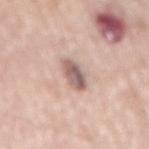Impression: This lesion was catalogued during total-body skin photography and was not selected for biopsy. Clinical summary: The lesion's longest dimension is about 4 mm. A 15 mm crop from a total-body photograph taken for skin-cancer surveillance. Automated image analysis of the tile measured an area of roughly 6 mm² and a shape eccentricity near 0.9. And it measured a lesion color around L≈60 a*≈17 b*≈22 in CIELAB and a normalized border contrast of about 9. The software also gave lesion-presence confidence of about 100/100. From the mid back. A male subject aged around 70.